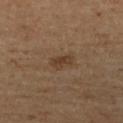  biopsy_status: not biopsied; imaged during a skin examination
  site: right lower leg
  patient:
    sex: male
    age_approx: 65
  lesion_size:
    long_diameter_mm_approx: 3.0
  image:
    source: total-body photography crop
    field_of_view_mm: 15
  automated_metrics:
    border_irregularity_0_10: 2.5
    color_variation_0_10: 1.5
    peripheral_color_asymmetry: 0.5